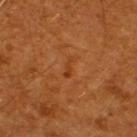This lesion was catalogued during total-body skin photography and was not selected for biopsy.
Imaged with cross-polarized lighting.
Located on the upper back.
A close-up tile cropped from a whole-body skin photograph, about 15 mm across.
About 2.5 mm across.
Automated image analysis of the tile measured a lesion color around L≈37 a*≈26 b*≈38 in CIELAB, roughly 6 lightness units darker than nearby skin, and a normalized lesion–skin contrast near 5.5. The analysis additionally found a border-irregularity index near 4.5/10, internal color variation of about 0 on a 0–10 scale, and a peripheral color-asymmetry measure near 0. The software also gave a classifier nevus-likeness of about 0/100 and lesion-presence confidence of about 100/100.
The patient is a male aged approximately 60.